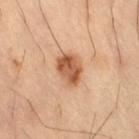<record>
<biopsy_status>not biopsied; imaged during a skin examination</biopsy_status>
<lesion_size>
  <long_diameter_mm_approx>3.5</long_diameter_mm_approx>
</lesion_size>
<image>
  <source>total-body photography crop</source>
  <field_of_view_mm>15</field_of_view_mm>
</image>
<site>right thigh</site>
<lighting>cross-polarized</lighting>
<patient>
  <sex>male</sex>
  <age_approx>60</age_approx>
</patient>
</record>